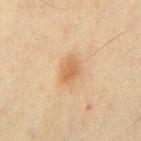Q: Was this lesion biopsied?
A: catalogued during a skin exam; not biopsied
Q: Patient demographics?
A: male, in their mid- to late 40s
Q: What is the imaging modality?
A: ~15 mm tile from a whole-body skin photo
Q: Lesion location?
A: the abdomen